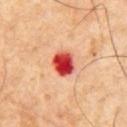Clinical impression:
Recorded during total-body skin imaging; not selected for excision or biopsy.
Acquisition and patient details:
A male subject, roughly 60 years of age. Located on the front of the torso. Cropped from a whole-body photographic skin survey; the tile spans about 15 mm.